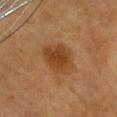Assessment: Part of a total-body skin-imaging series; this lesion was reviewed on a skin check and was not flagged for biopsy. Clinical summary: Approximately 4 mm at its widest. The subject is a female aged 63 to 67. This is a cross-polarized tile. Cropped from a whole-body photographic skin survey; the tile spans about 15 mm. The lesion is on the head or neck. An algorithmic analysis of the crop reported an automated nevus-likeness rating near 100 out of 100 and lesion-presence confidence of about 100/100.